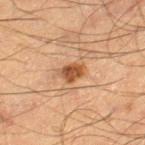No biopsy was performed on this lesion — it was imaged during a full skin examination and was not determined to be concerning. The lesion is on the leg. This is a cross-polarized tile. Cropped from a whole-body photographic skin survey; the tile spans about 15 mm. The total-body-photography lesion software estimated a footprint of about 4.5 mm² and a shape-asymmetry score of about 0.25 (0 = symmetric). The analysis additionally found roughly 12 lightness units darker than nearby skin. A male patient, aged 63 to 67. The lesion's longest dimension is about 3 mm.